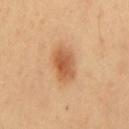workup = no biopsy performed (imaged during a skin exam) | anatomic site = the mid back | tile lighting = cross-polarized illumination | patient = male, about 50 years old | imaging modality = ~15 mm crop, total-body skin-cancer survey | size = ~4 mm (longest diameter).